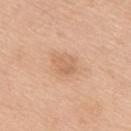biopsy status=imaged on a skin check; not biopsied | anatomic site=the upper back | patient=female, approximately 55 years of age | acquisition=15 mm crop, total-body photography.